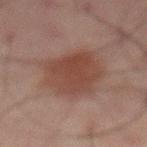No biopsy was performed on this lesion — it was imaged during a full skin examination and was not determined to be concerning. The lesion is located on the mid back. A 15 mm crop from a total-body photograph taken for skin-cancer surveillance. Automated tile analysis of the lesion measured a footprint of about 20 mm² and an eccentricity of roughly 0.5. It also reported an average lesion color of about L≈34 a*≈17 b*≈21 (CIELAB), roughly 7 lightness units darker than nearby skin, and a lesion-to-skin contrast of about 7 (normalized; higher = more distinct). And it measured border irregularity of about 2 on a 0–10 scale, a color-variation rating of about 2/10, and peripheral color asymmetry of about 0.5. The software also gave a nevus-likeness score of about 100/100 and a detector confidence of about 100 out of 100 that the crop contains a lesion. Imaged with cross-polarized lighting. A male subject, about 65 years old. The lesion's longest dimension is about 5 mm.